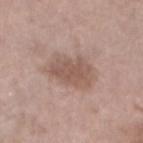Part of a total-body skin-imaging series; this lesion was reviewed on a skin check and was not flagged for biopsy.